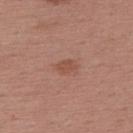Context: An algorithmic analysis of the crop reported a lesion area of about 3.5 mm² and a shape eccentricity near 0.8. The analysis additionally found a lesion–skin lightness drop of about 7 and a lesion-to-skin contrast of about 6 (normalized; higher = more distinct). The analysis additionally found a border-irregularity rating of about 2.5/10, internal color variation of about 0.5 on a 0–10 scale, and radial color variation of about 0. The analysis additionally found a nevus-likeness score of about 30/100 and a detector confidence of about 100 out of 100 that the crop contains a lesion. A 15 mm close-up extracted from a 3D total-body photography capture. A female subject about 55 years old. Longest diameter approximately 2.5 mm. From the upper back.